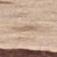lesion size: ~4 mm (longest diameter) | image-analysis metrics: a lesion area of about 4 mm², a shape eccentricity near 0.95, and a shape-asymmetry score of about 0.2 (0 = symmetric); radial color variation of about 0.5 | patient: male, aged approximately 70 | illumination: white-light | anatomic site: the right thigh | image source: ~15 mm crop, total-body skin-cancer survey.